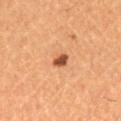follow-up: imaged on a skin check; not biopsied
site: the right lower leg
lighting: cross-polarized illumination
patient: female, aged approximately 30
acquisition: ~15 mm crop, total-body skin-cancer survey